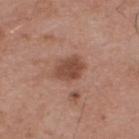Findings:
– follow-up: no biopsy performed (imaged during a skin exam)
– tile lighting: white-light illumination
– image: total-body-photography crop, ~15 mm field of view
– lesion size: about 4 mm
– patient: male, in their mid- to late 50s
– body site: the upper back